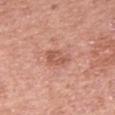Part of a total-body skin-imaging series; this lesion was reviewed on a skin check and was not flagged for biopsy. A female patient, aged approximately 40. A 15 mm close-up extracted from a 3D total-body photography capture. Imaged with white-light lighting. About 3.5 mm across. Automated image analysis of the tile measured a lesion color around L≈57 a*≈26 b*≈30 in CIELAB, about 9 CIELAB-L* units darker than the surrounding skin, and a normalized lesion–skin contrast near 6. And it measured a color-variation rating of about 2/10 and peripheral color asymmetry of about 0.5. From the right forearm.